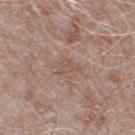Assessment:
Part of a total-body skin-imaging series; this lesion was reviewed on a skin check and was not flagged for biopsy.
Image and clinical context:
A male patient, aged 73 to 77. This is a white-light tile. A roughly 15 mm field-of-view crop from a total-body skin photograph. The lesion is on the right thigh.